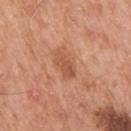The lesion was photographed on a routine skin check and not biopsied; there is no pathology result. The lesion is on the right upper arm. Measured at roughly 3.5 mm in maximum diameter. A region of skin cropped from a whole-body photographic capture, roughly 15 mm wide. A male subject aged around 55.